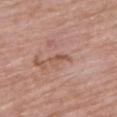The lesion was tiled from a total-body skin photograph and was not biopsied. The lesion is located on the upper back. The patient is a male approximately 85 years of age or older. A roughly 15 mm field-of-view crop from a total-body skin photograph.